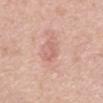Q: Was a biopsy performed?
A: catalogued during a skin exam; not biopsied
Q: Illumination type?
A: white-light
Q: How large is the lesion?
A: about 3 mm
Q: Who is the patient?
A: male, aged approximately 80
Q: How was this image acquired?
A: total-body-photography crop, ~15 mm field of view
Q: Lesion location?
A: the abdomen